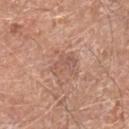Part of a total-body skin-imaging series; this lesion was reviewed on a skin check and was not flagged for biopsy.
A close-up tile cropped from a whole-body skin photograph, about 15 mm across.
A male subject roughly 80 years of age.
The lesion is on the right lower leg.